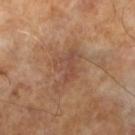| key | value |
|---|---|
| follow-up | no biopsy performed (imaged during a skin exam) |
| imaging modality | ~15 mm tile from a whole-body skin photo |
| lighting | cross-polarized |
| patient | male, in their mid-60s |
| automated lesion analysis | an area of roughly 9.5 mm² and a symmetry-axis asymmetry near 0.45; about 7 CIELAB-L* units darker than the surrounding skin and a lesion-to-skin contrast of about 5.5 (normalized; higher = more distinct); an automated nevus-likeness rating near 0 out of 100 and a detector confidence of about 95 out of 100 that the crop contains a lesion |
| lesion diameter | about 5 mm |